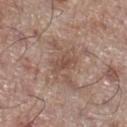subject = male, aged 68–72
image = ~15 mm tile from a whole-body skin photo
illumination = white-light
body site = the right lower leg